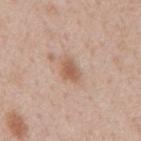Imaged during a routine full-body skin examination; the lesion was not biopsied and no histopathology is available. Approximately 3 mm at its widest. The patient is a male aged approximately 50. This image is a 15 mm lesion crop taken from a total-body photograph. The lesion is located on the back.